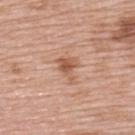The patient is a female aged 58 to 62.
The lesion is on the upper back.
An algorithmic analysis of the crop reported a footprint of about 5 mm² and a shape eccentricity near 0.6.
Longest diameter approximately 3 mm.
This is a white-light tile.
A close-up tile cropped from a whole-body skin photograph, about 15 mm across.